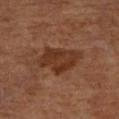workup: no biopsy performed (imaged during a skin exam)
acquisition: total-body-photography crop, ~15 mm field of view
anatomic site: the right lower leg
automated lesion analysis: a shape eccentricity near 0.7 and a shape-asymmetry score of about 0.3 (0 = symmetric); a classifier nevus-likeness of about 5/100
illumination: cross-polarized
subject: aged around 70